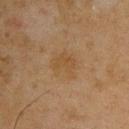Part of a total-body skin-imaging series; this lesion was reviewed on a skin check and was not flagged for biopsy.
A 15 mm crop from a total-body photograph taken for skin-cancer surveillance.
A male patient in their mid-40s.
Measured at roughly 4 mm in maximum diameter.
From the upper back.
The tile uses cross-polarized illumination.